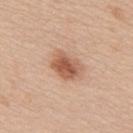Notes:
• illumination — white-light
• body site — the upper back
• TBP lesion metrics — an area of roughly 8 mm² and an eccentricity of roughly 0.7; a lesion-detection confidence of about 100/100
• patient — male, aged 63–67
• imaging modality — 15 mm crop, total-body photography
• size — about 3.5 mm